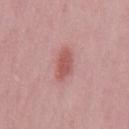The lesion was tiled from a total-body skin photograph and was not biopsied.
Longest diameter approximately 3.5 mm.
Cropped from a whole-body photographic skin survey; the tile spans about 15 mm.
On the mid back.
Imaged with white-light lighting.
A male patient aged approximately 50.